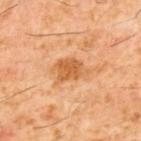Clinical impression: Part of a total-body skin-imaging series; this lesion was reviewed on a skin check and was not flagged for biopsy. Clinical summary: On the upper back. A roughly 15 mm field-of-view crop from a total-body skin photograph. A male patient, aged approximately 60. Approximately 4 mm at its widest. The tile uses cross-polarized illumination.